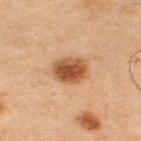| key | value |
|---|---|
| workup | no biopsy performed (imaged during a skin exam) |
| patient | male, roughly 65 years of age |
| image | total-body-photography crop, ~15 mm field of view |
| body site | the leg |
| lesion size | about 3.5 mm |